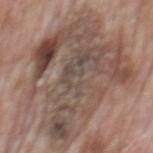workup: no biopsy performed (imaged during a skin exam) | body site: the mid back | subject: male, about 70 years old | automated lesion analysis: a shape eccentricity near 0.5 and a shape-asymmetry score of about 0.35 (0 = symmetric); border irregularity of about 5.5 on a 0–10 scale, a within-lesion color-variation index near 9/10, and radial color variation of about 3; a nevus-likeness score of about 0/100 and lesion-presence confidence of about 75/100 | acquisition: total-body-photography crop, ~15 mm field of view.